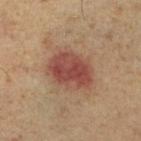image: ~15 mm crop, total-body skin-cancer survey | body site: the right lower leg | patient: male, in their mid-50s | size: ~5.5 mm (longest diameter).